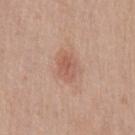Assessment: The lesion was tiled from a total-body skin photograph and was not biopsied. Background: Longest diameter approximately 3 mm. A region of skin cropped from a whole-body photographic capture, roughly 15 mm wide. The subject is a male aged 63 to 67. This is a white-light tile. An algorithmic analysis of the crop reported an outline eccentricity of about 0.7 (0 = round, 1 = elongated) and a shape-asymmetry score of about 0.2 (0 = symmetric). And it measured a lesion color around L≈57 a*≈23 b*≈29 in CIELAB. The analysis additionally found border irregularity of about 2 on a 0–10 scale and internal color variation of about 3 on a 0–10 scale. The analysis additionally found an automated nevus-likeness rating near 80 out of 100 and a lesion-detection confidence of about 100/100. On the chest.